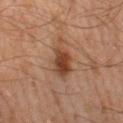biopsy status — imaged on a skin check; not biopsied
illumination — cross-polarized
image — ~15 mm crop, total-body skin-cancer survey
patient — male, in their mid-40s
location — the left lower leg